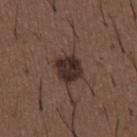Impression:
Recorded during total-body skin imaging; not selected for excision or biopsy.
Clinical summary:
A region of skin cropped from a whole-body photographic capture, roughly 15 mm wide. The tile uses white-light illumination. Approximately 4 mm at its widest. From the front of the torso. Automated image analysis of the tile measured an outline eccentricity of about 0.65 (0 = round, 1 = elongated). The analysis additionally found a border-irregularity rating of about 2/10. A male subject roughly 50 years of age.